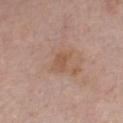| key | value |
|---|---|
| follow-up | catalogued during a skin exam; not biopsied |